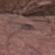Findings:
* workup: total-body-photography surveillance lesion; no biopsy
* TBP lesion metrics: border irregularity of about 3.5 on a 0–10 scale, internal color variation of about 4 on a 0–10 scale, and peripheral color asymmetry of about 1.5
* lighting: white-light illumination
* anatomic site: the left thigh
* lesion diameter: ~4 mm (longest diameter)
* subject: male, aged approximately 30
* image: 15 mm crop, total-body photography